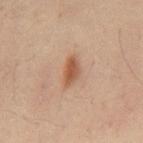No biopsy was performed on this lesion — it was imaged during a full skin examination and was not determined to be concerning. Located on the back. This image is a 15 mm lesion crop taken from a total-body photograph. A male subject roughly 50 years of age. The tile uses cross-polarized illumination.